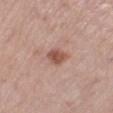The lesion was tiled from a total-body skin photograph and was not biopsied. The patient is a female roughly 65 years of age. The lesion is on the right lower leg. Imaged with white-light lighting. A 15 mm close-up extracted from a 3D total-body photography capture. Longest diameter approximately 2.5 mm. Automated tile analysis of the lesion measured a lesion area of about 4.5 mm² and an outline eccentricity of about 0.6 (0 = round, 1 = elongated). It also reported an average lesion color of about L≈53 a*≈23 b*≈27 (CIELAB) and a normalized lesion–skin contrast near 8.5. It also reported an automated nevus-likeness rating near 85 out of 100 and a lesion-detection confidence of about 100/100.